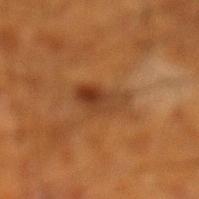• biopsy status: catalogued during a skin exam; not biopsied
• tile lighting: cross-polarized
• body site: the leg
• acquisition: ~15 mm tile from a whole-body skin photo
• patient: male, roughly 60 years of age